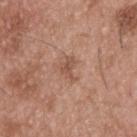The lesion was tiled from a total-body skin photograph and was not biopsied. A close-up tile cropped from a whole-body skin photograph, about 15 mm across. The lesion is located on the upper back. A male patient aged approximately 55. The lesion's longest dimension is about 3 mm. This is a white-light tile.